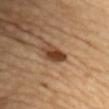  biopsy_status: not biopsied; imaged during a skin examination
  automated_metrics:
    area_mm2_approx: 5.5
    eccentricity: 0.85
    shape_asymmetry: 0.25
  lighting: cross-polarized
  site: chest
  patient:
    sex: female
    age_approx: 70
  image:
    source: total-body photography crop
    field_of_view_mm: 15